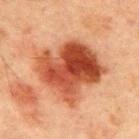Cropped from a total-body skin-imaging series; the visible field is about 15 mm. Located on the chest. Imaged with cross-polarized lighting. Measured at roughly 7.5 mm in maximum diameter. A male patient, in their mid- to late 60s.